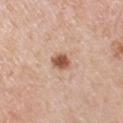The lesion was tiled from a total-body skin photograph and was not biopsied.
Approximately 2.5 mm at its widest.
Automated image analysis of the tile measured a mean CIELAB color near L≈55 a*≈22 b*≈32 and a normalized border contrast of about 10.
A 15 mm crop from a total-body photograph taken for skin-cancer surveillance.
The lesion is located on the arm.
A male subject aged 48 to 52.